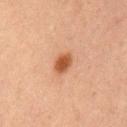Q: Was a biopsy performed?
A: imaged on a skin check; not biopsied
Q: Who is the patient?
A: male, aged around 65
Q: What is the anatomic site?
A: the mid back
Q: Illumination type?
A: cross-polarized
Q: What did automated image analysis measure?
A: a footprint of about 4.5 mm², an eccentricity of roughly 0.7, and a symmetry-axis asymmetry near 0.2; a classifier nevus-likeness of about 100/100 and lesion-presence confidence of about 100/100
Q: What kind of image is this?
A: ~15 mm tile from a whole-body skin photo